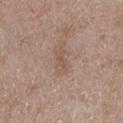Background:
A 15 mm close-up tile from a total-body photography series done for melanoma screening. The total-body-photography lesion software estimated an average lesion color of about L≈53 a*≈16 b*≈26 (CIELAB), a lesion–skin lightness drop of about 6, and a normalized lesion–skin contrast near 5. The analysis additionally found a classifier nevus-likeness of about 0/100 and a detector confidence of about 100 out of 100 that the crop contains a lesion. Longest diameter approximately 3 mm. A male patient aged around 50. From the leg. The tile uses white-light illumination.